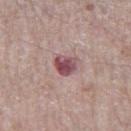follow-up: imaged on a skin check; not biopsied | patient: male, in their 70s | image: ~15 mm tile from a whole-body skin photo | site: the right thigh.